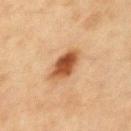Q: Was this lesion biopsied?
A: imaged on a skin check; not biopsied